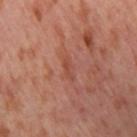notes=no biopsy performed (imaged during a skin exam); subject=female, in their mid- to late 50s; lighting=cross-polarized; image=total-body-photography crop, ~15 mm field of view; body site=the leg.